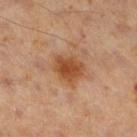The lesion was photographed on a routine skin check and not biopsied; there is no pathology result.
Automated image analysis of the tile measured about 10 CIELAB-L* units darker than the surrounding skin and a lesion-to-skin contrast of about 9 (normalized; higher = more distinct). The analysis additionally found border irregularity of about 2 on a 0–10 scale and radial color variation of about 1. It also reported a nevus-likeness score of about 85/100 and lesion-presence confidence of about 100/100.
Captured under cross-polarized illumination.
A male patient in their 60s.
Longest diameter approximately 3.5 mm.
A 15 mm close-up tile from a total-body photography series done for melanoma screening.
On the right thigh.